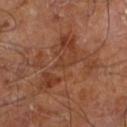Q: Was this lesion biopsied?
A: imaged on a skin check; not biopsied
Q: Where on the body is the lesion?
A: the right leg
Q: What is the lesion's diameter?
A: about 8 mm
Q: What are the patient's age and sex?
A: male, aged around 60
Q: What lighting was used for the tile?
A: cross-polarized
Q: What did automated image analysis measure?
A: a footprint of about 23 mm², a shape eccentricity near 0.75, and two-axis asymmetry of about 0.65; a mean CIELAB color near L≈38 a*≈22 b*≈30, roughly 7 lightness units darker than nearby skin, and a lesion-to-skin contrast of about 6 (normalized; higher = more distinct)
Q: What is the imaging modality?
A: 15 mm crop, total-body photography